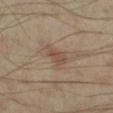| key | value |
|---|---|
| workup | catalogued during a skin exam; not biopsied |
| lighting | cross-polarized illumination |
| subject | male, aged around 55 |
| lesion size | ≈3.5 mm |
| image-analysis metrics | a footprint of about 5 mm² and an eccentricity of roughly 0.8; an average lesion color of about L≈49 a*≈15 b*≈28 (CIELAB) and a normalized lesion–skin contrast near 6; a nevus-likeness score of about 5/100 and lesion-presence confidence of about 100/100 |
| imaging modality | 15 mm crop, total-body photography |
| body site | the left lower leg |